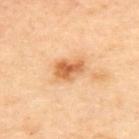  biopsy_status: not biopsied; imaged during a skin examination
  image:
    source: total-body photography crop
    field_of_view_mm: 15
  patient:
    sex: female
    age_approx: 40
  site: upper back
  lesion_size:
    long_diameter_mm_approx: 3.5